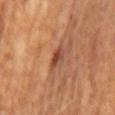Impression:
The lesion was tiled from a total-body skin photograph and was not biopsied.
Image and clinical context:
A 15 mm close-up tile from a total-body photography series done for melanoma screening. This is a cross-polarized tile. The patient is a male roughly 65 years of age. Longest diameter approximately 2.5 mm. Automated image analysis of the tile measured a lesion area of about 3.5 mm² and a shape-asymmetry score of about 0.35 (0 = symmetric). The software also gave a border-irregularity index near 3/10 and a within-lesion color-variation index near 4/10.